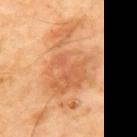{
  "biopsy_status": "not biopsied; imaged during a skin examination",
  "automated_metrics": {
    "cielab_L": 63,
    "cielab_a": 25,
    "cielab_b": 41,
    "vs_skin_darker_L": 10.0,
    "vs_skin_contrast_norm": 6.5,
    "border_irregularity_0_10": 6.5,
    "color_variation_0_10": 5.5,
    "peripheral_color_asymmetry": 1.5
  },
  "site": "mid back",
  "lesion_size": {
    "long_diameter_mm_approx": 10.5
  },
  "lighting": "cross-polarized",
  "image": {
    "source": "total-body photography crop",
    "field_of_view_mm": 15
  },
  "patient": {
    "sex": "male",
    "age_approx": 65
  }
}